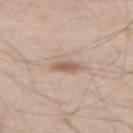workup: total-body-photography surveillance lesion; no biopsy.